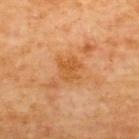No biopsy was performed on this lesion — it was imaged during a full skin examination and was not determined to be concerning. A 15 mm crop from a total-body photograph taken for skin-cancer surveillance. The lesion is located on the upper back. A male patient about 60 years old.